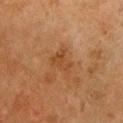notes: total-body-photography surveillance lesion; no biopsy
tile lighting: cross-polarized
image source: total-body-photography crop, ~15 mm field of view
subject: female, in their 50s
automated lesion analysis: a mean CIELAB color near L≈37 a*≈20 b*≈32 and about 6 CIELAB-L* units darker than the surrounding skin; a border-irregularity rating of about 5/10, a within-lesion color-variation index near 2/10, and a peripheral color-asymmetry measure near 0.5; a classifier nevus-likeness of about 0/100 and a detector confidence of about 100 out of 100 that the crop contains a lesion
lesion diameter: about 3 mm
anatomic site: the chest